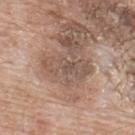Q: What is the anatomic site?
A: the upper back
Q: What are the patient's age and sex?
A: male, aged approximately 65
Q: Illumination type?
A: white-light
Q: What kind of image is this?
A: 15 mm crop, total-body photography
Q: Lesion size?
A: ≈6 mm